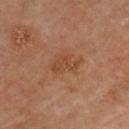site: chest
patient:
  sex: male
  age_approx: 65
lesion_size:
  long_diameter_mm_approx: 3.0
lighting: cross-polarized
image:
  source: total-body photography crop
  field_of_view_mm: 15